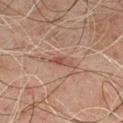Recorded during total-body skin imaging; not selected for excision or biopsy. The recorded lesion diameter is about 3 mm. Captured under cross-polarized illumination. A male subject aged around 45. A roughly 15 mm field-of-view crop from a total-body skin photograph. An algorithmic analysis of the crop reported an average lesion color of about L≈40 a*≈19 b*≈23 (CIELAB), about 7 CIELAB-L* units darker than the surrounding skin, and a normalized lesion–skin contrast near 6.5. It also reported a border-irregularity index near 3.5/10 and a peripheral color-asymmetry measure near 0. The software also gave a nevus-likeness score of about 30/100 and a detector confidence of about 100 out of 100 that the crop contains a lesion. The lesion is located on the chest.